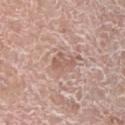The lesion was photographed on a routine skin check and not biopsied; there is no pathology result.
A male subject, roughly 75 years of age.
Located on the left lower leg.
Automated image analysis of the tile measured an area of roughly 4 mm², a shape eccentricity near 0.85, and two-axis asymmetry of about 0.55.
A roughly 15 mm field-of-view crop from a total-body skin photograph.
Captured under white-light illumination.